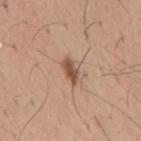Clinical impression: The lesion was tiled from a total-body skin photograph and was not biopsied. Context: Automated tile analysis of the lesion measured internal color variation of about 2.5 on a 0–10 scale and a peripheral color-asymmetry measure near 0.5. And it measured an automated nevus-likeness rating near 85 out of 100 and a detector confidence of about 100 out of 100 that the crop contains a lesion. The patient is a male aged approximately 65. A lesion tile, about 15 mm wide, cut from a 3D total-body photograph. Captured under white-light illumination. Located on the mid back. Longest diameter approximately 3 mm.